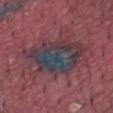Part of a total-body skin-imaging series; this lesion was reviewed on a skin check and was not flagged for biopsy. Captured under white-light illumination. Measured at roughly 8.5 mm in maximum diameter. Located on the arm. This image is a 15 mm lesion crop taken from a total-body photograph. The subject is a male about 40 years old.